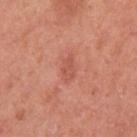<record>
<biopsy_status>not biopsied; imaged during a skin examination</biopsy_status>
<lesion_size>
  <long_diameter_mm_approx>3.5</long_diameter_mm_approx>
</lesion_size>
<patient>
  <sex>female</sex>
  <age_approx>40</age_approx>
</patient>
<lighting>white-light</lighting>
<site>left upper arm</site>
<image>
  <source>total-body photography crop</source>
  <field_of_view_mm>15</field_of_view_mm>
</image>
</record>